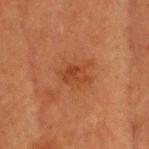Assessment: Part of a total-body skin-imaging series; this lesion was reviewed on a skin check and was not flagged for biopsy. Clinical summary: The subject is a male aged around 75. The tile uses cross-polarized illumination. The lesion is on the head or neck. Automated image analysis of the tile measured roughly 7 lightness units darker than nearby skin and a normalized border contrast of about 6. The analysis additionally found a border-irregularity rating of about 6/10, internal color variation of about 1 on a 0–10 scale, and a peripheral color-asymmetry measure near 0. About 3 mm across. Cropped from a total-body skin-imaging series; the visible field is about 15 mm.